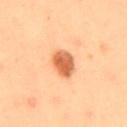Recorded during total-body skin imaging; not selected for excision or biopsy. From the mid back. Measured at roughly 3.5 mm in maximum diameter. Captured under cross-polarized illumination. Automated image analysis of the tile measured an eccentricity of roughly 0.7 and a shape-asymmetry score of about 0.1 (0 = symmetric). It also reported a nevus-likeness score of about 100/100 and lesion-presence confidence of about 100/100. The patient is a female aged approximately 40. A 15 mm close-up tile from a total-body photography series done for melanoma screening.